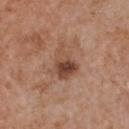workup=imaged on a skin check; not biopsied
imaging modality=~15 mm tile from a whole-body skin photo
subject=female, about 65 years old
location=the chest